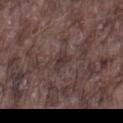Assessment: Imaged during a routine full-body skin examination; the lesion was not biopsied and no histopathology is available. Clinical summary: A male subject in their mid-70s. A 15 mm close-up tile from a total-body photography series done for melanoma screening. From the right thigh.